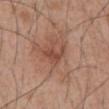Imaged during a routine full-body skin examination; the lesion was not biopsied and no histopathology is available. On the abdomen. A close-up tile cropped from a whole-body skin photograph, about 15 mm across. A male patient roughly 55 years of age.